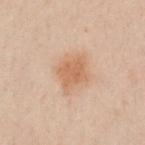Notes:
– workup: total-body-photography surveillance lesion; no biopsy
– image-analysis metrics: an average lesion color of about L≈50 a*≈17 b*≈28 (CIELAB), about 7 CIELAB-L* units darker than the surrounding skin, and a lesion-to-skin contrast of about 6.5 (normalized; higher = more distinct); a nevus-likeness score of about 85/100 and lesion-presence confidence of about 100/100
– subject: male, approximately 50 years of age
– tile lighting: cross-polarized illumination
– size: ≈3.5 mm
– body site: the arm
– acquisition: ~15 mm tile from a whole-body skin photo